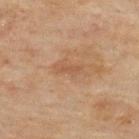Recorded during total-body skin imaging; not selected for excision or biopsy.
A lesion tile, about 15 mm wide, cut from a 3D total-body photograph.
Measured at roughly 2.5 mm in maximum diameter.
A male subject in their mid-40s.
Captured under cross-polarized illumination.
The lesion is on the upper back.